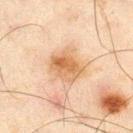Context: Longest diameter approximately 4 mm. A 15 mm close-up tile from a total-body photography series done for melanoma screening. The tile uses cross-polarized illumination. A male patient approximately 45 years of age. From the right thigh.